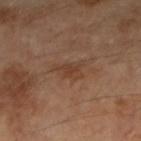Findings:
- follow-up — imaged on a skin check; not biopsied
- subject — female, roughly 60 years of age
- illumination — cross-polarized illumination
- imaging modality — total-body-photography crop, ~15 mm field of view
- body site — the right forearm